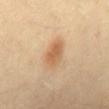Clinical impression:
The lesion was tiled from a total-body skin photograph and was not biopsied.
Acquisition and patient details:
On the abdomen. A region of skin cropped from a whole-body photographic capture, roughly 15 mm wide. A male patient, approximately 40 years of age.